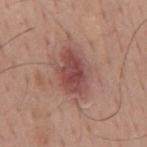An algorithmic analysis of the crop reported a mean CIELAB color near L≈48 a*≈24 b*≈23, roughly 11 lightness units darker than nearby skin, and a lesion-to-skin contrast of about 8 (normalized; higher = more distinct). The analysis additionally found a nevus-likeness score of about 70/100 and lesion-presence confidence of about 100/100. A lesion tile, about 15 mm wide, cut from a 3D total-body photograph. The lesion is on the mid back. A male patient about 60 years old.